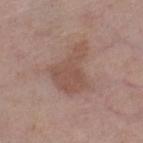The lesion was tiled from a total-body skin photograph and was not biopsied. Automated tile analysis of the lesion measured an area of roughly 16 mm² and an eccentricity of roughly 0.7. The software also gave a mean CIELAB color near L≈51 a*≈19 b*≈25, about 8 CIELAB-L* units darker than the surrounding skin, and a normalized border contrast of about 6. The analysis additionally found an automated nevus-likeness rating near 0 out of 100 and a lesion-detection confidence of about 100/100. Imaged with white-light lighting. A roughly 15 mm field-of-view crop from a total-body skin photograph. A female subject in their mid- to late 60s. On the right lower leg. The recorded lesion diameter is about 6 mm.